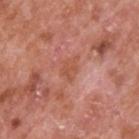  lighting: white-light
  site: upper back
  image:
    source: total-body photography crop
    field_of_view_mm: 15
  patient:
    sex: male
    age_approx: 65